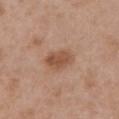Impression: Imaged during a routine full-body skin examination; the lesion was not biopsied and no histopathology is available. Background: The recorded lesion diameter is about 3.5 mm. An algorithmic analysis of the crop reported a mean CIELAB color near L≈51 a*≈21 b*≈31 and roughly 10 lightness units darker than nearby skin. Captured under white-light illumination. The lesion is located on the chest. The patient is a female aged around 40. Cropped from a whole-body photographic skin survey; the tile spans about 15 mm.